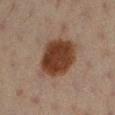<case>
  <biopsy_status>not biopsied; imaged during a skin examination</biopsy_status>
  <patient>
    <sex>female</sex>
    <age_approx>20</age_approx>
  </patient>
  <lesion_size>
    <long_diameter_mm_approx>6.0</long_diameter_mm_approx>
  </lesion_size>
  <lighting>cross-polarized</lighting>
  <automated_metrics>
    <eccentricity>0.65</eccentricity>
    <shape_asymmetry>0.15</shape_asymmetry>
    <color_variation_0_10>3.5</color_variation_0_10>
    <peripheral_color_asymmetry>1.0</peripheral_color_asymmetry>
    <nevus_likeness_0_100>100</nevus_likeness_0_100>
    <lesion_detection_confidence_0_100>100</lesion_detection_confidence_0_100>
  </automated_metrics>
  <image>
    <source>total-body photography crop</source>
    <field_of_view_mm>15</field_of_view_mm>
  </image>
  <site>leg</site>
</case>